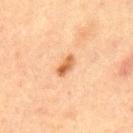Imaged with cross-polarized lighting.
Longest diameter approximately 3 mm.
Cropped from a total-body skin-imaging series; the visible field is about 15 mm.
A male patient, about 65 years old.
The lesion is located on the front of the torso.
The lesion-visualizer software estimated a lesion area of about 3.5 mm², an outline eccentricity of about 0.9 (0 = round, 1 = elongated), and a symmetry-axis asymmetry near 0.25. And it measured a lesion color around L≈51 a*≈21 b*≈35 in CIELAB.